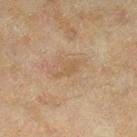The lesion was photographed on a routine skin check and not biopsied; there is no pathology result. A 15 mm close-up tile from a total-body photography series done for melanoma screening. The tile uses cross-polarized illumination. The lesion is on the right lower leg. The total-body-photography lesion software estimated a mean CIELAB color near L≈51 a*≈15 b*≈33, about 6 CIELAB-L* units darker than the surrounding skin, and a normalized border contrast of about 5. The software also gave an automated nevus-likeness rating near 0 out of 100 and lesion-presence confidence of about 100/100. A female patient aged 58–62.